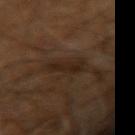The total-body-photography lesion software estimated a lesion area of about 5.5 mm² and a symmetry-axis asymmetry near 0.35. The analysis additionally found a within-lesion color-variation index near 1.5/10 and radial color variation of about 0.5. And it measured an automated nevus-likeness rating near 0 out of 100 and lesion-presence confidence of about 75/100.
The lesion's longest dimension is about 4 mm.
This is a cross-polarized tile.
A male subject, aged approximately 60.
On the right arm.
This image is a 15 mm lesion crop taken from a total-body photograph.